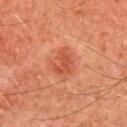<case>
<biopsy_status>not biopsied; imaged during a skin examination</biopsy_status>
<site>chest</site>
<lesion_size>
  <long_diameter_mm_approx>3.5</long_diameter_mm_approx>
</lesion_size>
<patient>
  <sex>male</sex>
  <age_approx>65</age_approx>
</patient>
<image>
  <source>total-body photography crop</source>
  <field_of_view_mm>15</field_of_view_mm>
</image>
<lighting>cross-polarized</lighting>
</case>